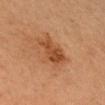  biopsy_status: not biopsied; imaged during a skin examination
  lighting: cross-polarized
  automated_metrics:
    cielab_L: 43
    cielab_a: 23
    cielab_b: 35
    vs_skin_darker_L: 9.0
    vs_skin_contrast_norm: 7.5
  patient:
    sex: male
    age_approx: 65
  site: head or neck
  image:
    source: total-body photography crop
    field_of_view_mm: 15